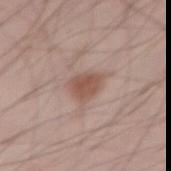{
  "biopsy_status": "not biopsied; imaged during a skin examination",
  "image": {
    "source": "total-body photography crop",
    "field_of_view_mm": 15
  },
  "patient": {
    "sex": "male",
    "age_approx": 55
  },
  "lesion_size": {
    "long_diameter_mm_approx": 3.0
  },
  "site": "right thigh",
  "automated_metrics": {
    "area_mm2_approx": 6.5,
    "eccentricity": 0.65,
    "shape_asymmetry": 0.25
  },
  "lighting": "white-light"
}